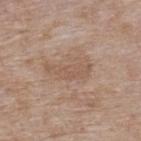biopsy status = no biopsy performed (imaged during a skin exam).